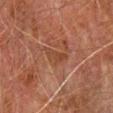biopsy status: no biopsy performed (imaged during a skin exam)
location: the left forearm
lesion diameter: ≈3 mm
TBP lesion metrics: an area of roughly 4.5 mm², a shape eccentricity near 0.7, and two-axis asymmetry of about 0.35; a lesion-detection confidence of about 95/100
subject: male, roughly 75 years of age
lighting: cross-polarized illumination
image: total-body-photography crop, ~15 mm field of view